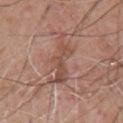  biopsy_status: not biopsied; imaged during a skin examination
  patient:
    sex: male
    age_approx: 60
  lesion_size:
    long_diameter_mm_approx: 6.5
  image:
    source: total-body photography crop
    field_of_view_mm: 15
  automated_metrics:
    area_mm2_approx: 9.5
    eccentricity: 0.95
    shape_asymmetry: 0.6
    cielab_L: 50
    cielab_a: 20
    cielab_b: 26
    vs_skin_darker_L: 8.0
    vs_skin_contrast_norm: 6.0
    border_irregularity_0_10: 9.5
    color_variation_0_10: 2.5
    peripheral_color_asymmetry: 1.0
    nevus_likeness_0_100: 0
    lesion_detection_confidence_0_100: 65
  site: chest